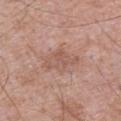Recorded during total-body skin imaging; not selected for excision or biopsy.
A close-up tile cropped from a whole-body skin photograph, about 15 mm across.
From the front of the torso.
This is a white-light tile.
A male patient aged 68 to 72.